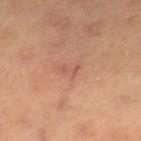Imaged during a routine full-body skin examination; the lesion was not biopsied and no histopathology is available. Automated tile analysis of the lesion measured an area of roughly 3 mm², an eccentricity of roughly 0.75, and a shape-asymmetry score of about 0.65 (0 = symmetric). It also reported a detector confidence of about 95 out of 100 that the crop contains a lesion. Located on the right lower leg. A roughly 15 mm field-of-view crop from a total-body skin photograph. This is a cross-polarized tile. About 2.5 mm across. A female patient, aged approximately 70.